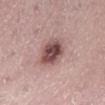Context: A female patient, approximately 25 years of age. A 15 mm crop from a total-body photograph taken for skin-cancer surveillance. The lesion is located on the left lower leg.